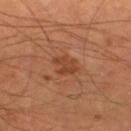Q: Was this lesion biopsied?
A: imaged on a skin check; not biopsied
Q: What is the imaging modality?
A: ~15 mm crop, total-body skin-cancer survey
Q: Who is the patient?
A: male, aged around 65
Q: Where on the body is the lesion?
A: the right thigh